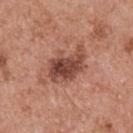Q: Is there a histopathology result?
A: no biopsy performed (imaged during a skin exam)
Q: Patient demographics?
A: male, about 55 years old
Q: What is the imaging modality?
A: total-body-photography crop, ~15 mm field of view
Q: What did automated image analysis measure?
A: border irregularity of about 4 on a 0–10 scale, a within-lesion color-variation index near 6/10, and a peripheral color-asymmetry measure near 2
Q: Where on the body is the lesion?
A: the upper back
Q: How was the tile lit?
A: white-light